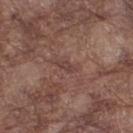Imaged during a routine full-body skin examination; the lesion was not biopsied and no histopathology is available. The lesion is located on the leg. The patient is a male in their mid- to late 70s. A lesion tile, about 15 mm wide, cut from a 3D total-body photograph.